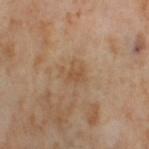Clinical impression: The lesion was tiled from a total-body skin photograph and was not biopsied. Background: The total-body-photography lesion software estimated a lesion area of about 4.5 mm² and two-axis asymmetry of about 0.3. The analysis additionally found an average lesion color of about L≈52 a*≈18 b*≈33 (CIELAB) and a normalized lesion–skin contrast near 5. The analysis additionally found a classifier nevus-likeness of about 0/100 and lesion-presence confidence of about 100/100. On the right thigh. A 15 mm crop from a total-body photograph taken for skin-cancer surveillance. A female patient, in their mid- to late 50s.